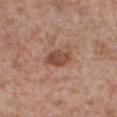workup = catalogued during a skin exam; not biopsied
size = ~3.5 mm (longest diameter)
anatomic site = the chest
subject = male, aged 63 to 67
illumination = white-light
image = ~15 mm crop, total-body skin-cancer survey